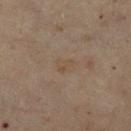Imaged during a routine full-body skin examination; the lesion was not biopsied and no histopathology is available.
A female patient roughly 60 years of age.
On the right lower leg.
The tile uses cross-polarized illumination.
The recorded lesion diameter is about 2.5 mm.
A region of skin cropped from a whole-body photographic capture, roughly 15 mm wide.